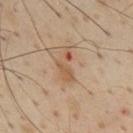The tile uses cross-polarized illumination. Automated image analysis of the tile measured a shape-asymmetry score of about 0.55 (0 = symmetric). The analysis additionally found a border-irregularity index near 7/10, a within-lesion color-variation index near 2/10, and a peripheral color-asymmetry measure near 0.5. On the front of the torso. A male patient, aged approximately 55. A roughly 15 mm field-of-view crop from a total-body skin photograph.